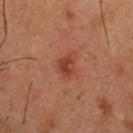{
  "biopsy_status": "not biopsied; imaged during a skin examination",
  "site": "chest",
  "patient": {
    "sex": "male",
    "age_approx": 50
  },
  "lighting": "cross-polarized",
  "image": {
    "source": "total-body photography crop",
    "field_of_view_mm": 15
  },
  "lesion_size": {
    "long_diameter_mm_approx": 3.0
  }
}